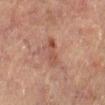workup: catalogued during a skin exam; not biopsied | body site: the left lower leg | image source: total-body-photography crop, ~15 mm field of view | diameter: ≈3.5 mm | illumination: cross-polarized | subject: female, approximately 70 years of age.